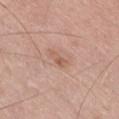Captured during whole-body skin photography for melanoma surveillance; the lesion was not biopsied.
A male patient roughly 75 years of age.
Longest diameter approximately 2.5 mm.
The lesion is on the right thigh.
Cropped from a whole-body photographic skin survey; the tile spans about 15 mm.